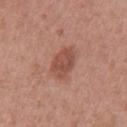No biopsy was performed on this lesion — it was imaged during a full skin examination and was not determined to be concerning.
The subject is a female approximately 30 years of age.
This image is a 15 mm lesion crop taken from a total-body photograph.
The tile uses white-light illumination.
The lesion is on the left upper arm.
The lesion's longest dimension is about 4 mm.